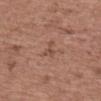Impression:
Recorded during total-body skin imaging; not selected for excision or biopsy.
Context:
About 3 mm across. A female subject in their mid-60s. Automated image analysis of the tile measured a border-irregularity rating of about 6.5/10, internal color variation of about 0 on a 0–10 scale, and radial color variation of about 0. The tile uses white-light illumination. The lesion is on the mid back. A 15 mm crop from a total-body photograph taken for skin-cancer surveillance.